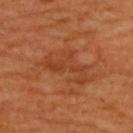Assessment:
The lesion was photographed on a routine skin check and not biopsied; there is no pathology result.
Acquisition and patient details:
A 15 mm close-up extracted from a 3D total-body photography capture. An algorithmic analysis of the crop reported a lesion–skin lightness drop of about 6 and a lesion-to-skin contrast of about 5 (normalized; higher = more distinct). It also reported a classifier nevus-likeness of about 0/100. The subject is a female in their mid-40s. On the back. The lesion's longest dimension is about 6.5 mm. Imaged with cross-polarized lighting.